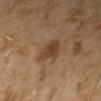| field | value |
|---|---|
| workup | no biopsy performed (imaged during a skin exam) |
| tile lighting | cross-polarized illumination |
| size | about 3 mm |
| automated lesion analysis | a lesion color around L≈35 a*≈16 b*≈28 in CIELAB, about 8 CIELAB-L* units darker than the surrounding skin, and a normalized lesion–skin contrast near 8; an automated nevus-likeness rating near 65 out of 100 and a detector confidence of about 100 out of 100 that the crop contains a lesion |
| patient | female, in their 60s |
| acquisition | ~15 mm crop, total-body skin-cancer survey |
| location | the left forearm |